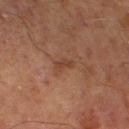Assessment: Imaged during a routine full-body skin examination; the lesion was not biopsied and no histopathology is available. Context: A male patient roughly 70 years of age. A roughly 15 mm field-of-view crop from a total-body skin photograph. The lesion's longest dimension is about 2.5 mm. On the left lower leg. This is a cross-polarized tile.